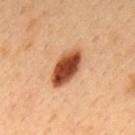Q: Was a biopsy performed?
A: total-body-photography surveillance lesion; no biopsy